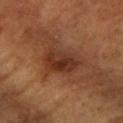Imaged during a routine full-body skin examination; the lesion was not biopsied and no histopathology is available. Automated tile analysis of the lesion measured border irregularity of about 2 on a 0–10 scale. The software also gave a lesion-detection confidence of about 100/100. On the left forearm. A 15 mm crop from a total-body photograph taken for skin-cancer surveillance. Captured under cross-polarized illumination. A female subject, aged 58 to 62. Approximately 5 mm at its widest.